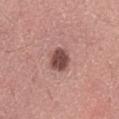Q: Was a biopsy performed?
A: catalogued during a skin exam; not biopsied
Q: What is the imaging modality?
A: 15 mm crop, total-body photography
Q: What did automated image analysis measure?
A: a lesion area of about 6.5 mm², an outline eccentricity of about 0.35 (0 = round, 1 = elongated), and a symmetry-axis asymmetry near 0.15; a lesion color around L≈47 a*≈22 b*≈23 in CIELAB, roughly 15 lightness units darker than nearby skin, and a normalized lesion–skin contrast near 10.5; a border-irregularity rating of about 1.5/10, internal color variation of about 2.5 on a 0–10 scale, and radial color variation of about 1
Q: Lesion location?
A: the lower back
Q: How large is the lesion?
A: about 3 mm
Q: What are the patient's age and sex?
A: male, aged 38 to 42
Q: Illumination type?
A: white-light